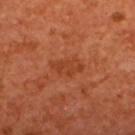  lesion_size:
    long_diameter_mm_approx: 3.5
  site: back
  lighting: cross-polarized
  image:
    source: total-body photography crop
    field_of_view_mm: 15
  patient:
    sex: female
    age_approx: 55